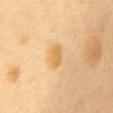Clinical impression: The lesion was photographed on a routine skin check and not biopsied; there is no pathology result. Clinical summary: Longest diameter approximately 3 mm. Located on the chest. A female subject approximately 50 years of age. A region of skin cropped from a whole-body photographic capture, roughly 15 mm wide. Imaged with cross-polarized lighting.